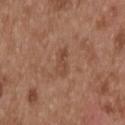Assessment: Imaged during a routine full-body skin examination; the lesion was not biopsied and no histopathology is available. Acquisition and patient details: A roughly 15 mm field-of-view crop from a total-body skin photograph. The tile uses white-light illumination. An algorithmic analysis of the crop reported a lesion color around L≈46 a*≈21 b*≈29 in CIELAB and about 7 CIELAB-L* units darker than the surrounding skin. It also reported internal color variation of about 3 on a 0–10 scale and radial color variation of about 1. And it measured an automated nevus-likeness rating near 0 out of 100 and a lesion-detection confidence of about 100/100. A male patient aged around 55. Longest diameter approximately 3 mm. Located on the back.